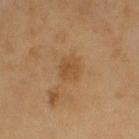Background: Captured under cross-polarized illumination. On the right upper arm. Cropped from a whole-body photographic skin survey; the tile spans about 15 mm. Longest diameter approximately 2.5 mm. A male patient aged 58 to 62.